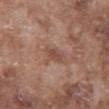Assessment: This lesion was catalogued during total-body skin photography and was not selected for biopsy. Background: The patient is a male in their mid- to late 70s. Located on the chest. This image is a 15 mm lesion crop taken from a total-body photograph.